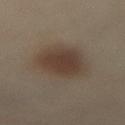Recorded during total-body skin imaging; not selected for excision or biopsy. The lesion is located on the lower back. Longest diameter approximately 6 mm. The subject is a female approximately 40 years of age. This is a cross-polarized tile. A 15 mm close-up extracted from a 3D total-body photography capture.